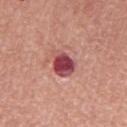workup: catalogued during a skin exam; not biopsied | acquisition: ~15 mm crop, total-body skin-cancer survey | patient: male, aged around 65 | lesion size: ~3.5 mm (longest diameter) | TBP lesion metrics: a footprint of about 7.5 mm² and an outline eccentricity of about 0.6 (0 = round, 1 = elongated); a mean CIELAB color near L≈47 a*≈33 b*≈21 and a lesion-to-skin contrast of about 12.5 (normalized; higher = more distinct); border irregularity of about 2.5 on a 0–10 scale, a color-variation rating of about 5/10, and peripheral color asymmetry of about 1.5; an automated nevus-likeness rating near 0 out of 100 and a detector confidence of about 100 out of 100 that the crop contains a lesion | body site: the chest | illumination: white-light.